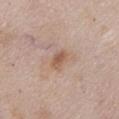Impression: Part of a total-body skin-imaging series; this lesion was reviewed on a skin check and was not flagged for biopsy. Background: From the chest. A female subject in their mid-70s. A 15 mm close-up tile from a total-body photography series done for melanoma screening.